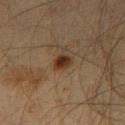Captured during whole-body skin photography for melanoma surveillance; the lesion was not biopsied. About 2 mm across. The tile uses cross-polarized illumination. A close-up tile cropped from a whole-body skin photograph, about 15 mm across. The total-body-photography lesion software estimated a lesion–skin lightness drop of about 10 and a normalized border contrast of about 11. And it measured border irregularity of about 2 on a 0–10 scale, a color-variation rating of about 3/10, and radial color variation of about 1. Located on the left thigh. The patient is a male aged around 60.